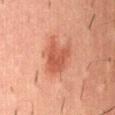Image and clinical context:
The tile uses cross-polarized illumination. The subject is a male aged 53–57. A lesion tile, about 15 mm wide, cut from a 3D total-body photograph. Measured at roughly 4.5 mm in maximum diameter. From the lower back.